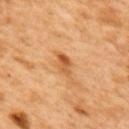Impression:
Part of a total-body skin-imaging series; this lesion was reviewed on a skin check and was not flagged for biopsy.
Clinical summary:
A male patient aged 48 to 52. A 15 mm crop from a total-body photograph taken for skin-cancer surveillance. From the back. The total-body-photography lesion software estimated an area of roughly 5 mm², a shape eccentricity near 0.85, and a shape-asymmetry score of about 0.25 (0 = symmetric). And it measured an average lesion color of about L≈58 a*≈25 b*≈44 (CIELAB) and a normalized border contrast of about 7.5. Captured under cross-polarized illumination.